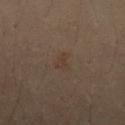{"biopsy_status": "not biopsied; imaged during a skin examination", "lighting": "cross-polarized", "automated_metrics": {"eccentricity": 0.8, "shape_asymmetry": 0.45, "border_irregularity_0_10": 4.5, "color_variation_0_10": 0.5}, "site": "lower back", "image": {"source": "total-body photography crop", "field_of_view_mm": 15}, "patient": {"sex": "male", "age_approx": 30}, "lesion_size": {"long_diameter_mm_approx": 2.5}}